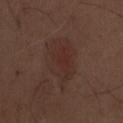Q: Was this lesion biopsied?
A: imaged on a skin check; not biopsied
Q: Automated lesion metrics?
A: an area of roughly 19 mm², an eccentricity of roughly 0.75, and a symmetry-axis asymmetry near 0.3; a lesion–skin lightness drop of about 5 and a normalized border contrast of about 5; radial color variation of about 1
Q: What kind of image is this?
A: ~15 mm tile from a whole-body skin photo
Q: Lesion size?
A: ≈6.5 mm
Q: What lighting was used for the tile?
A: white-light
Q: What is the anatomic site?
A: the front of the torso
Q: Patient demographics?
A: male, in their 70s